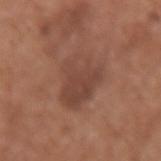{"biopsy_status": "not biopsied; imaged during a skin examination", "image": {"source": "total-body photography crop", "field_of_view_mm": 15}, "site": "arm", "lesion_size": {"long_diameter_mm_approx": 5.0}, "lighting": "white-light", "patient": {"sex": "female", "age_approx": 50}}